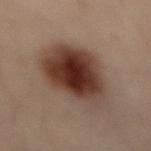Recorded during total-body skin imaging; not selected for excision or biopsy. A male subject aged 48 to 52. Measured at roughly 8 mm in maximum diameter. The lesion is located on the lower back. This image is a 15 mm lesion crop taken from a total-body photograph. The tile uses cross-polarized illumination.